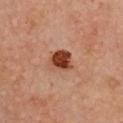Clinical impression:
Recorded during total-body skin imaging; not selected for excision or biopsy.
Background:
A 15 mm close-up tile from a total-body photography series done for melanoma screening. The lesion is on the chest. A female subject aged approximately 35.